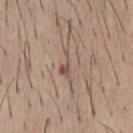<record>
<biopsy_status>not biopsied; imaged during a skin examination</biopsy_status>
<site>chest</site>
<automated_metrics>
  <area_mm2_approx>4.0</area_mm2_approx>
  <eccentricity>0.95</eccentricity>
  <shape_asymmetry>0.6</shape_asymmetry>
  <cielab_L>53</cielab_L>
  <cielab_a>17</cielab_a>
  <cielab_b>23</cielab_b>
  <vs_skin_darker_L>9.0</vs_skin_darker_L>
  <vs_skin_contrast_norm>6.5</vs_skin_contrast_norm>
</automated_metrics>
<image>
  <source>total-body photography crop</source>
  <field_of_view_mm>15</field_of_view_mm>
</image>
<lesion_size>
  <long_diameter_mm_approx>4.0</long_diameter_mm_approx>
</lesion_size>
<lighting>white-light</lighting>
<patient>
  <sex>male</sex>
  <age_approx>60</age_approx>
</patient>
</record>